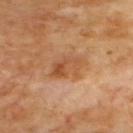No biopsy was performed on this lesion — it was imaged during a full skin examination and was not determined to be concerning.
On the upper back.
Measured at roughly 4.5 mm in maximum diameter.
The patient is a male aged around 70.
An algorithmic analysis of the crop reported a nevus-likeness score of about 10/100.
A close-up tile cropped from a whole-body skin photograph, about 15 mm across.
Imaged with cross-polarized lighting.